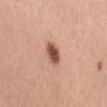{"biopsy_status": "not biopsied; imaged during a skin examination", "site": "lower back", "lighting": "white-light", "image": {"source": "total-body photography crop", "field_of_view_mm": 15}, "lesion_size": {"long_diameter_mm_approx": 2.5}, "automated_metrics": {"cielab_L": 52, "cielab_a": 24, "cielab_b": 29, "vs_skin_contrast_norm": 11.0, "border_irregularity_0_10": 2.0, "color_variation_0_10": 3.0, "peripheral_color_asymmetry": 1.0, "nevus_likeness_0_100": 100}, "patient": {"sex": "female", "age_approx": 30}}